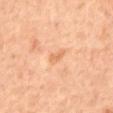Q: Is there a histopathology result?
A: imaged on a skin check; not biopsied